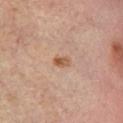Notes:
• biopsy status — imaged on a skin check; not biopsied
• anatomic site — the right lower leg
• subject — male, aged approximately 45
• automated lesion analysis — an area of roughly 2.5 mm², an outline eccentricity of about 0.8 (0 = round, 1 = elongated), and a shape-asymmetry score of about 0.2 (0 = symmetric); an average lesion color of about L≈50 a*≈20 b*≈30 (CIELAB), about 10 CIELAB-L* units darker than the surrounding skin, and a normalized border contrast of about 8; border irregularity of about 2 on a 0–10 scale, internal color variation of about 5 on a 0–10 scale, and a peripheral color-asymmetry measure near 2
• lesion size — ≈2 mm
• imaging modality — 15 mm crop, total-body photography
• illumination — cross-polarized